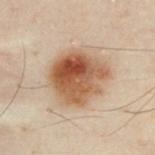  biopsy_status: not biopsied; imaged during a skin examination
  patient:
    sex: male
    age_approx: 50
  site: chest
  image:
    source: total-body photography crop
    field_of_view_mm: 15
  lesion_size:
    long_diameter_mm_approx: 6.0